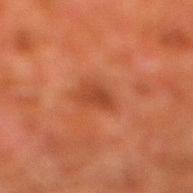Recorded during total-body skin imaging; not selected for excision or biopsy. Measured at roughly 3 mm in maximum diameter. A roughly 15 mm field-of-view crop from a total-body skin photograph. A male patient, aged 78–82. Located on the right lower leg. Imaged with cross-polarized lighting.